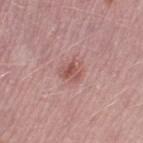biopsy_status: not biopsied; imaged during a skin examination
automated_metrics:
  cielab_L: 54
  cielab_a: 24
  cielab_b: 23
  vs_skin_darker_L: 9.0
  vs_skin_contrast_norm: 6.5
  nevus_likeness_0_100: 65
  lesion_detection_confidence_0_100: 100
lighting: white-light
patient:
  sex: male
  age_approx: 50
site: leg
image:
  source: total-body photography crop
  field_of_view_mm: 15